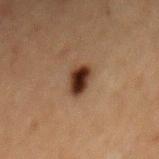Located on the mid back. A roughly 15 mm field-of-view crop from a total-body skin photograph. A female subject approximately 60 years of age.